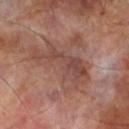The lesion was photographed on a routine skin check and not biopsied; there is no pathology result.
Captured under cross-polarized illumination.
The lesion-visualizer software estimated an average lesion color of about L≈44 a*≈21 b*≈24 (CIELAB), roughly 8 lightness units darker than nearby skin, and a lesion-to-skin contrast of about 6 (normalized; higher = more distinct). And it measured an automated nevus-likeness rating near 0 out of 100 and a detector confidence of about 95 out of 100 that the crop contains a lesion.
A male subject, aged approximately 70.
Measured at roughly 7.5 mm in maximum diameter.
The lesion is located on the left lower leg.
A region of skin cropped from a whole-body photographic capture, roughly 15 mm wide.